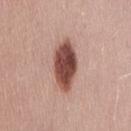Q: Is there a histopathology result?
A: total-body-photography surveillance lesion; no biopsy
Q: What kind of image is this?
A: 15 mm crop, total-body photography
Q: Patient demographics?
A: female, aged approximately 35
Q: How large is the lesion?
A: ~6 mm (longest diameter)
Q: Illumination type?
A: white-light illumination
Q: What is the anatomic site?
A: the abdomen
Q: Automated lesion metrics?
A: a lesion area of about 14 mm², a shape eccentricity near 0.85, and a symmetry-axis asymmetry near 0.1; a border-irregularity index near 1.5/10, a within-lesion color-variation index near 5.5/10, and radial color variation of about 1.5; a nevus-likeness score of about 100/100 and a lesion-detection confidence of about 100/100